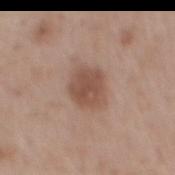Context:
A roughly 15 mm field-of-view crop from a total-body skin photograph. The patient is a male in their mid-50s. The recorded lesion diameter is about 4 mm. The lesion-visualizer software estimated a footprint of about 10 mm², an outline eccentricity of about 0.55 (0 = round, 1 = elongated), and a shape-asymmetry score of about 0.2 (0 = symmetric). And it measured an average lesion color of about L≈50 a*≈19 b*≈27 (CIELAB), about 10 CIELAB-L* units darker than the surrounding skin, and a lesion-to-skin contrast of about 7.5 (normalized; higher = more distinct). The software also gave a classifier nevus-likeness of about 85/100. On the mid back. Captured under white-light illumination.